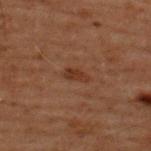patient = male, about 60 years old | imaging modality = ~15 mm crop, total-body skin-cancer survey | anatomic site = the upper back.